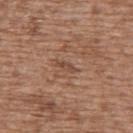Impression:
Captured during whole-body skin photography for melanoma surveillance; the lesion was not biopsied.
Clinical summary:
The patient is a female about 75 years old. Measured at roughly 2.5 mm in maximum diameter. A 15 mm close-up tile from a total-body photography series done for melanoma screening. Located on the upper back.